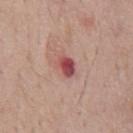This lesion was catalogued during total-body skin photography and was not selected for biopsy. The lesion's longest dimension is about 3 mm. A close-up tile cropped from a whole-body skin photograph, about 15 mm across. A male subject, approximately 70 years of age. The lesion is on the chest. Captured under white-light illumination.